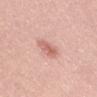Assessment:
This lesion was catalogued during total-body skin photography and was not selected for biopsy.
Clinical summary:
A female patient aged 53 to 57. The recorded lesion diameter is about 3.5 mm. Located on the mid back. Cropped from a whole-body photographic skin survey; the tile spans about 15 mm. Imaged with white-light lighting.